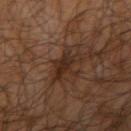{
  "lesion_size": {
    "long_diameter_mm_approx": 4.0
  },
  "lighting": "cross-polarized",
  "automated_metrics": {
    "vs_skin_darker_L": 7.0,
    "vs_skin_contrast_norm": 8.0,
    "border_irregularity_0_10": 7.5,
    "color_variation_0_10": 3.0,
    "peripheral_color_asymmetry": 1.0,
    "nevus_likeness_0_100": 5
  },
  "site": "right upper arm",
  "image": {
    "source": "total-body photography crop",
    "field_of_view_mm": 15
  },
  "patient": {
    "sex": "male",
    "age_approx": 65
  }
}